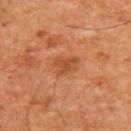follow-up: catalogued during a skin exam; not biopsied | lighting: cross-polarized illumination | imaging modality: ~15 mm tile from a whole-body skin photo | subject: male, aged 58–62 | size: about 3 mm | site: the front of the torso.